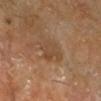notes = imaged on a skin check; not biopsied
illumination = cross-polarized
subject = male, aged around 60
automated lesion analysis = a lesion area of about 8 mm², a shape eccentricity near 0.7, and two-axis asymmetry of about 0.2; a classifier nevus-likeness of about 0/100
site = the right lower leg
lesion size = ~3.5 mm (longest diameter)
imaging modality = 15 mm crop, total-body photography